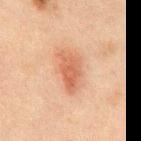Impression: The lesion was photographed on a routine skin check and not biopsied; there is no pathology result. Context: On the abdomen. Cropped from a whole-body photographic skin survey; the tile spans about 15 mm. A male patient aged approximately 50.